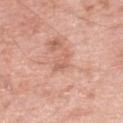follow-up = imaged on a skin check; not biopsied | automated metrics = a footprint of about 4 mm², a shape eccentricity near 0.8, and two-axis asymmetry of about 0.3; a border-irregularity rating of about 3/10, internal color variation of about 1.5 on a 0–10 scale, and radial color variation of about 0.5; a lesion-detection confidence of about 100/100 | patient = male, aged around 80 | lesion diameter = ≈3 mm | imaging modality = 15 mm crop, total-body photography | lighting = white-light | location = the right lower leg.